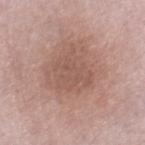Q: Was this lesion biopsied?
A: catalogued during a skin exam; not biopsied
Q: What is the lesion's diameter?
A: about 5 mm
Q: What is the anatomic site?
A: the right lower leg
Q: Who is the patient?
A: male, about 65 years old
Q: What lighting was used for the tile?
A: white-light
Q: How was this image acquired?
A: ~15 mm tile from a whole-body skin photo